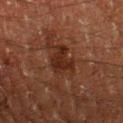{"biopsy_status": "not biopsied; imaged during a skin examination"}